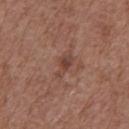The lesion was tiled from a total-body skin photograph and was not biopsied.
From the chest.
A male patient, approximately 75 years of age.
A 15 mm crop from a total-body photograph taken for skin-cancer surveillance.
Imaged with white-light lighting.
Approximately 3 mm at its widest.
Automated tile analysis of the lesion measured a mean CIELAB color near L≈43 a*≈20 b*≈25 and a normalized border contrast of about 6.5. And it measured border irregularity of about 4.5 on a 0–10 scale, internal color variation of about 3 on a 0–10 scale, and peripheral color asymmetry of about 1. It also reported a nevus-likeness score of about 20/100 and a lesion-detection confidence of about 100/100.